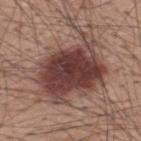Recorded during total-body skin imaging; not selected for excision or biopsy.
Imaged with white-light lighting.
The recorded lesion diameter is about 9.5 mm.
A 15 mm close-up extracted from a 3D total-body photography capture.
The lesion is on the back.
A male patient, approximately 60 years of age.
Automated image analysis of the tile measured border irregularity of about 6.5 on a 0–10 scale, a color-variation rating of about 6.5/10, and peripheral color asymmetry of about 2. The software also gave an automated nevus-likeness rating near 85 out of 100 and lesion-presence confidence of about 100/100.